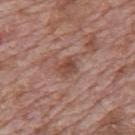Captured during whole-body skin photography for melanoma surveillance; the lesion was not biopsied. Captured under white-light illumination. Automated tile analysis of the lesion measured a lesion color around L≈47 a*≈22 b*≈26 in CIELAB, roughly 9 lightness units darker than nearby skin, and a lesion-to-skin contrast of about 7 (normalized; higher = more distinct). And it measured border irregularity of about 3.5 on a 0–10 scale, a color-variation rating of about 4/10, and radial color variation of about 1.5. A 15 mm close-up tile from a total-body photography series done for melanoma screening. Located on the mid back. About 3 mm across. A male patient, aged around 70.